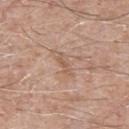Context:
Measured at roughly 3 mm in maximum diameter. An algorithmic analysis of the crop reported an average lesion color of about L≈57 a*≈18 b*≈30 (CIELAB). The software also gave a border-irregularity rating of about 4.5/10, internal color variation of about 0 on a 0–10 scale, and a peripheral color-asymmetry measure near 0. Located on the front of the torso. Captured under white-light illumination. A male subject, aged 63–67. A region of skin cropped from a whole-body photographic capture, roughly 15 mm wide.